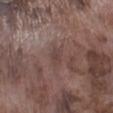No biopsy was performed on this lesion — it was imaged during a full skin examination and was not determined to be concerning.
From the left lower leg.
Imaged with white-light lighting.
A roughly 15 mm field-of-view crop from a total-body skin photograph.
A male patient roughly 75 years of age.